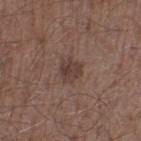No biopsy was performed on this lesion — it was imaged during a full skin examination and was not determined to be concerning. Measured at roughly 3 mm in maximum diameter. An algorithmic analysis of the crop reported a footprint of about 5.5 mm², a shape eccentricity near 0.45, and a shape-asymmetry score of about 0.2 (0 = symmetric). The analysis additionally found about 8 CIELAB-L* units darker than the surrounding skin and a normalized lesion–skin contrast near 7.5. The analysis additionally found border irregularity of about 2 on a 0–10 scale and peripheral color asymmetry of about 1. A lesion tile, about 15 mm wide, cut from a 3D total-body photograph. The tile uses white-light illumination. Located on the left thigh. A male subject aged 53 to 57.